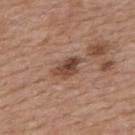Q: Was this lesion biopsied?
A: imaged on a skin check; not biopsied
Q: Where on the body is the lesion?
A: the upper back
Q: Who is the patient?
A: female, about 60 years old
Q: How large is the lesion?
A: ~3.5 mm (longest diameter)
Q: What lighting was used for the tile?
A: white-light illumination
Q: What kind of image is this?
A: ~15 mm crop, total-body skin-cancer survey